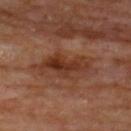Impression:
No biopsy was performed on this lesion — it was imaged during a full skin examination and was not determined to be concerning.
Context:
A lesion tile, about 15 mm wide, cut from a 3D total-body photograph. The recorded lesion diameter is about 6.5 mm. Automated tile analysis of the lesion measured an automated nevus-likeness rating near 10 out of 100 and lesion-presence confidence of about 100/100. The lesion is located on the upper back. The subject is a male approximately 70 years of age. Captured under cross-polarized illumination.